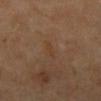Background: The subject is in their mid- to late 60s. Imaged with cross-polarized lighting. Approximately 3 mm at its widest. The lesion is located on the mid back. Cropped from a total-body skin-imaging series; the visible field is about 15 mm. The lesion-visualizer software estimated a lesion area of about 1.5 mm², an outline eccentricity of about 0.95 (0 = round, 1 = elongated), and a symmetry-axis asymmetry near 0.6.